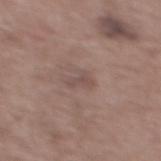Clinical impression: The lesion was photographed on a routine skin check and not biopsied; there is no pathology result. Acquisition and patient details: Located on the mid back. The subject is a male roughly 60 years of age. A 15 mm close-up tile from a total-body photography series done for melanoma screening.